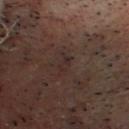Q: Was this lesion biopsied?
A: no biopsy performed (imaged during a skin exam)
Q: Lesion location?
A: the head or neck
Q: What are the patient's age and sex?
A: male, in their mid- to late 60s
Q: What did automated image analysis measure?
A: a lesion area of about 3 mm² and two-axis asymmetry of about 0.45; a mean CIELAB color near L≈24 a*≈13 b*≈16 and a lesion-to-skin contrast of about 5.5 (normalized; higher = more distinct); border irregularity of about 5 on a 0–10 scale, a color-variation rating of about 0/10, and radial color variation of about 0; a nevus-likeness score of about 0/100 and a detector confidence of about 85 out of 100 that the crop contains a lesion
Q: How was the tile lit?
A: cross-polarized
Q: How large is the lesion?
A: ≈3 mm
Q: How was this image acquired?
A: 15 mm crop, total-body photography